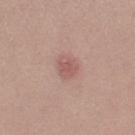Clinical impression: The lesion was tiled from a total-body skin photograph and was not biopsied. Clinical summary: Measured at roughly 2.5 mm in maximum diameter. The patient is a female aged approximately 20. A close-up tile cropped from a whole-body skin photograph, about 15 mm across. On the right thigh.